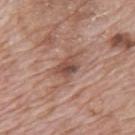No biopsy was performed on this lesion — it was imaged during a full skin examination and was not determined to be concerning.
The subject is a male approximately 70 years of age.
Captured under white-light illumination.
A lesion tile, about 15 mm wide, cut from a 3D total-body photograph.
Located on the mid back.